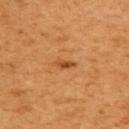Q: Was a biopsy performed?
A: no biopsy performed (imaged during a skin exam)
Q: Where on the body is the lesion?
A: the back
Q: Who is the patient?
A: female, roughly 55 years of age
Q: What kind of image is this?
A: total-body-photography crop, ~15 mm field of view
Q: Lesion size?
A: ~2.5 mm (longest diameter)
Q: What lighting was used for the tile?
A: cross-polarized illumination